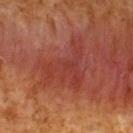workup: catalogued during a skin exam; not biopsied | anatomic site: the upper back | lesion diameter: ≈7 mm | subject: male, in their 60s | acquisition: ~15 mm tile from a whole-body skin photo | illumination: cross-polarized | automated metrics: a shape eccentricity near 0.65 and a symmetry-axis asymmetry near 0.55; a mean CIELAB color near L≈33 a*≈26 b*≈25, about 5 CIELAB-L* units darker than the surrounding skin, and a normalized lesion–skin contrast near 5; a classifier nevus-likeness of about 0/100 and a lesion-detection confidence of about 100/100.